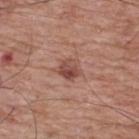notes=imaged on a skin check; not biopsied
subject=male, aged 68–72
tile lighting=white-light illumination
image=total-body-photography crop, ~15 mm field of view
body site=the upper back
diameter=~2.5 mm (longest diameter)